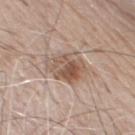Imaged during a routine full-body skin examination; the lesion was not biopsied and no histopathology is available.
A 15 mm crop from a total-body photograph taken for skin-cancer surveillance.
On the front of the torso.
Captured under white-light illumination.
The subject is a male aged around 80.